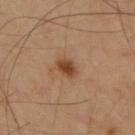Part of a total-body skin-imaging series; this lesion was reviewed on a skin check and was not flagged for biopsy. A roughly 15 mm field-of-view crop from a total-body skin photograph. The lesion is located on the upper back. A male subject in their mid- to late 60s.